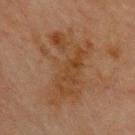Clinical impression: This lesion was catalogued during total-body skin photography and was not selected for biopsy. Image and clinical context: This is a cross-polarized tile. The lesion is on the chest. Longest diameter approximately 9 mm. The subject is a male aged around 70. This image is a 15 mm lesion crop taken from a total-body photograph.